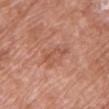Q: Is there a histopathology result?
A: total-body-photography surveillance lesion; no biopsy
Q: Lesion size?
A: about 4 mm
Q: Patient demographics?
A: female, aged 68–72
Q: What kind of image is this?
A: ~15 mm tile from a whole-body skin photo
Q: Automated lesion metrics?
A: a footprint of about 3.5 mm², an outline eccentricity of about 0.95 (0 = round, 1 = elongated), and a shape-asymmetry score of about 0.6 (0 = symmetric); about 7 CIELAB-L* units darker than the surrounding skin; border irregularity of about 8 on a 0–10 scale, a color-variation rating of about 0/10, and peripheral color asymmetry of about 0; a nevus-likeness score of about 0/100 and a detector confidence of about 100 out of 100 that the crop contains a lesion
Q: Where on the body is the lesion?
A: the chest